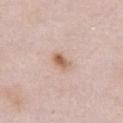notes — imaged on a skin check; not biopsied | lesion size — ~2.5 mm (longest diameter) | subject — female, roughly 65 years of age | acquisition — 15 mm crop, total-body photography | anatomic site — the chest | image-analysis metrics — a footprint of about 3.5 mm², an outline eccentricity of about 0.8 (0 = round, 1 = elongated), and two-axis asymmetry of about 0.2; a lesion color around L≈62 a*≈19 b*≈30 in CIELAB and a lesion-to-skin contrast of about 8.5 (normalized; higher = more distinct) | lighting — white-light.